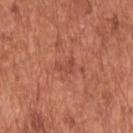The lesion was photographed on a routine skin check and not biopsied; there is no pathology result. From the back. Imaged with white-light lighting. A 15 mm close-up extracted from a 3D total-body photography capture. The patient is a male aged 63–67. Measured at roughly 2.5 mm in maximum diameter.